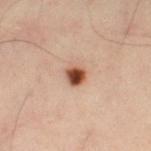Assessment: Part of a total-body skin-imaging series; this lesion was reviewed on a skin check and was not flagged for biopsy. Context: Measured at roughly 2.5 mm in maximum diameter. A male patient, roughly 40 years of age. A lesion tile, about 15 mm wide, cut from a 3D total-body photograph. From the leg.